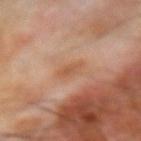Imaged during a routine full-body skin examination; the lesion was not biopsied and no histopathology is available.
On the arm.
A 15 mm crop from a total-body photograph taken for skin-cancer surveillance.
Imaged with cross-polarized lighting.
The lesion's longest dimension is about 3 mm.
The lesion-visualizer software estimated an eccentricity of roughly 0.85 and a shape-asymmetry score of about 0.3 (0 = symmetric). The analysis additionally found a border-irregularity index near 3/10, a within-lesion color-variation index near 2.5/10, and radial color variation of about 1. The software also gave a detector confidence of about 100 out of 100 that the crop contains a lesion.
A male patient, about 70 years old.